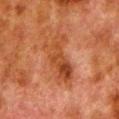Cropped from a whole-body photographic skin survey; the tile spans about 15 mm. Imaged with cross-polarized lighting. Measured at roughly 6 mm in maximum diameter. On the left lower leg. A male patient, aged 78 to 82. An algorithmic analysis of the crop reported a lesion–skin lightness drop of about 7 and a lesion-to-skin contrast of about 7 (normalized; higher = more distinct). The analysis additionally found a color-variation rating of about 7/10 and peripheral color asymmetry of about 2.5.